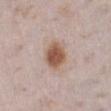Case summary:
– site: the left lower leg
– size: about 4 mm
– tile lighting: white-light illumination
– image: 15 mm crop, total-body photography
– patient: female, about 30 years old